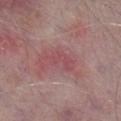Assessment:
Part of a total-body skin-imaging series; this lesion was reviewed on a skin check and was not flagged for biopsy.
Clinical summary:
A male patient, aged 73 to 77. Located on the leg. Captured under white-light illumination. A roughly 15 mm field-of-view crop from a total-body skin photograph.